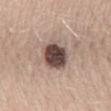Findings:
• workup: no biopsy performed (imaged during a skin exam)
• lighting: white-light illumination
• patient: female, in their mid-40s
• imaging modality: ~15 mm crop, total-body skin-cancer survey
• body site: the mid back
• lesion diameter: ≈4 mm
• automated metrics: roughly 20 lightness units darker than nearby skin and a normalized border contrast of about 13.5; border irregularity of about 1 on a 0–10 scale and internal color variation of about 6 on a 0–10 scale; a lesion-detection confidence of about 100/100